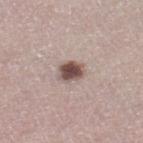This lesion was catalogued during total-body skin photography and was not selected for biopsy. A female subject, in their mid-50s. A region of skin cropped from a whole-body photographic capture, roughly 15 mm wide. Captured under white-light illumination. An algorithmic analysis of the crop reported a lesion area of about 5.5 mm², an outline eccentricity of about 0.6 (0 = round, 1 = elongated), and two-axis asymmetry of about 0.25. The analysis additionally found a lesion color around L≈50 a*≈16 b*≈21 in CIELAB, roughly 17 lightness units darker than nearby skin, and a lesion-to-skin contrast of about 11.5 (normalized; higher = more distinct). And it measured border irregularity of about 2 on a 0–10 scale, a within-lesion color-variation index near 3.5/10, and radial color variation of about 1. The software also gave a nevus-likeness score of about 95/100 and a lesion-detection confidence of about 100/100. Longest diameter approximately 3 mm. On the right lower leg.